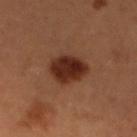Part of a total-body skin-imaging series; this lesion was reviewed on a skin check and was not flagged for biopsy. The subject is a female roughly 45 years of age. A close-up tile cropped from a whole-body skin photograph, about 15 mm across. Located on the leg.